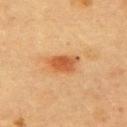Case summary:
• workup — no biopsy performed (imaged during a skin exam)
• illumination — cross-polarized
• image-analysis metrics — a footprint of about 8.5 mm² and an outline eccentricity of about 0.8 (0 = round, 1 = elongated); a border-irregularity index near 2/10, internal color variation of about 4 on a 0–10 scale, and radial color variation of about 1.5
• image — ~15 mm tile from a whole-body skin photo
• subject — female, aged 58 to 62
• diameter — ~4 mm (longest diameter)
• anatomic site — the upper back